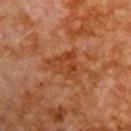Imaged during a routine full-body skin examination; the lesion was not biopsied and no histopathology is available. Automated tile analysis of the lesion measured an average lesion color of about L≈33 a*≈22 b*≈31 (CIELAB) and roughly 5 lightness units darker than nearby skin. The software also gave a border-irregularity rating of about 5.5/10 and peripheral color asymmetry of about 1. And it measured a classifier nevus-likeness of about 0/100 and lesion-presence confidence of about 100/100. This image is a 15 mm lesion crop taken from a total-body photograph. The subject is a male aged 78 to 82. On the chest. This is a cross-polarized tile.